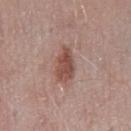| feature | finding |
|---|---|
| biopsy status | catalogued during a skin exam; not biopsied |
| anatomic site | the back |
| subject | male, about 55 years old |
| illumination | white-light illumination |
| imaging modality | 15 mm crop, total-body photography |
| automated lesion analysis | an area of roughly 8 mm² and an outline eccentricity of about 0.85 (0 = round, 1 = elongated); a border-irregularity rating of about 3/10, internal color variation of about 3.5 on a 0–10 scale, and a peripheral color-asymmetry measure near 1 |
| diameter | about 4.5 mm |